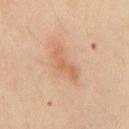The lesion was tiled from a total-body skin photograph and was not biopsied. The lesion is located on the chest. The lesion's longest dimension is about 4 mm. A male patient aged 48 to 52. Captured under cross-polarized illumination. An algorithmic analysis of the crop reported a lesion area of about 5 mm², an eccentricity of roughly 0.9, and a symmetry-axis asymmetry near 0.55. The software also gave a lesion–skin lightness drop of about 8 and a normalized border contrast of about 5.5. And it measured border irregularity of about 6.5 on a 0–10 scale, a within-lesion color-variation index near 0.5/10, and a peripheral color-asymmetry measure near 0. It also reported an automated nevus-likeness rating near 0 out of 100 and lesion-presence confidence of about 100/100. A 15 mm crop from a total-body photograph taken for skin-cancer surveillance.